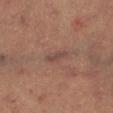<case>
  <patient>
    <sex>female</sex>
    <age_approx>60</age_approx>
  </patient>
  <lesion_size>
    <long_diameter_mm_approx>3.0</long_diameter_mm_approx>
  </lesion_size>
  <site>left thigh</site>
  <lighting>cross-polarized</lighting>
  <image>
    <source>total-body photography crop</source>
    <field_of_view_mm>15</field_of_view_mm>
  </image>
</case>